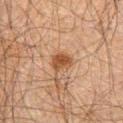Assessment:
Captured during whole-body skin photography for melanoma surveillance; the lesion was not biopsied.
Background:
On the chest. A close-up tile cropped from a whole-body skin photograph, about 15 mm across. The lesion-visualizer software estimated a shape eccentricity near 0.65 and two-axis asymmetry of about 0.25. The software also gave a color-variation rating of about 2/10 and peripheral color asymmetry of about 0.5. It also reported a classifier nevus-likeness of about 90/100 and lesion-presence confidence of about 100/100. The tile uses cross-polarized illumination. The recorded lesion diameter is about 3 mm. A male patient, aged 58–62.